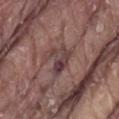workup: catalogued during a skin exam; not biopsied
TBP lesion metrics: a lesion color around L≈40 a*≈18 b*≈17 in CIELAB and a lesion-to-skin contrast of about 7.5 (normalized; higher = more distinct); a border-irregularity rating of about 6/10, internal color variation of about 8.5 on a 0–10 scale, and radial color variation of about 2.5; a classifier nevus-likeness of about 0/100 and lesion-presence confidence of about 65/100
location: the lower back
tile lighting: white-light
image: 15 mm crop, total-body photography
lesion size: about 3.5 mm
subject: male, aged 78–82